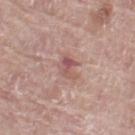* workup — catalogued during a skin exam; not biopsied
* diameter — about 3 mm
* body site — the left thigh
* illumination — white-light illumination
* imaging modality — ~15 mm tile from a whole-body skin photo
* patient — female, about 60 years old